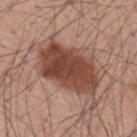Captured during whole-body skin photography for melanoma surveillance; the lesion was not biopsied. The lesion is on the back. A male subject, about 60 years old. A region of skin cropped from a whole-body photographic capture, roughly 15 mm wide.